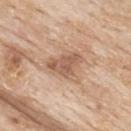No biopsy was performed on this lesion — it was imaged during a full skin examination and was not determined to be concerning.